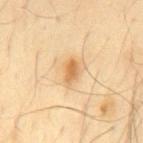– imaging modality · 15 mm crop, total-body photography
– automated metrics · border irregularity of about 3 on a 0–10 scale, internal color variation of about 3.5 on a 0–10 scale, and peripheral color asymmetry of about 1
– diameter · about 3 mm
– illumination · cross-polarized
– anatomic site · the mid back
– subject · male, roughly 65 years of age